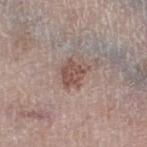Imaged during a routine full-body skin examination; the lesion was not biopsied and no histopathology is available. About 3.5 mm across. A close-up tile cropped from a whole-body skin photograph, about 15 mm across. A male subject about 80 years old. The tile uses white-light illumination. Located on the left thigh.